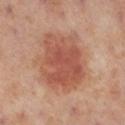Recorded during total-body skin imaging; not selected for excision or biopsy. A 15 mm close-up extracted from a 3D total-body photography capture. A female patient in their mid- to late 50s. Located on the right lower leg.